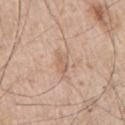Q: Illumination type?
A: white-light illumination
Q: What are the patient's age and sex?
A: male, aged approximately 65
Q: How large is the lesion?
A: ≈3 mm
Q: Automated lesion metrics?
A: a detector confidence of about 95 out of 100 that the crop contains a lesion
Q: Lesion location?
A: the right upper arm
Q: What kind of image is this?
A: ~15 mm crop, total-body skin-cancer survey